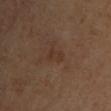Captured during whole-body skin photography for melanoma surveillance; the lesion was not biopsied. An algorithmic analysis of the crop reported a lesion area of about 3.5 mm² and two-axis asymmetry of about 0.3. And it measured border irregularity of about 3 on a 0–10 scale, a within-lesion color-variation index near 1.5/10, and peripheral color asymmetry of about 0.5. And it measured a classifier nevus-likeness of about 0/100 and a detector confidence of about 100 out of 100 that the crop contains a lesion. The lesion's longest dimension is about 3 mm. A female subject aged around 40. Located on the front of the torso. A 15 mm close-up extracted from a 3D total-body photography capture.